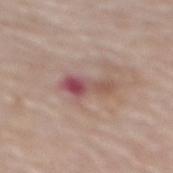{"biopsy_status": "not biopsied; imaged during a skin examination", "site": "mid back", "patient": {"sex": "male", "age_approx": 75}, "automated_metrics": {"cielab_L": 51, "cielab_a": 23, "cielab_b": 21, "vs_skin_contrast_norm": 8.5, "border_irregularity_0_10": 5.5, "color_variation_0_10": 9.0, "peripheral_color_asymmetry": 2.5, "nevus_likeness_0_100": 0, "lesion_detection_confidence_0_100": 100}, "lighting": "white-light", "image": {"source": "total-body photography crop", "field_of_view_mm": 15}}